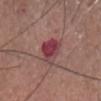{
  "biopsy_status": "not biopsied; imaged during a skin examination",
  "image": {
    "source": "total-body photography crop",
    "field_of_view_mm": 15
  },
  "lesion_size": {
    "long_diameter_mm_approx": 4.0
  },
  "automated_metrics": {
    "vs_skin_contrast_norm": 9.5,
    "border_irregularity_0_10": 3.0,
    "color_variation_0_10": 7.0
  },
  "patient": {
    "sex": "male",
    "age_approx": 55
  },
  "lighting": "white-light",
  "site": "head or neck"
}